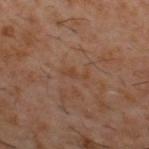Findings:
• follow-up — catalogued during a skin exam; not biopsied
• patient — male, approximately 60 years of age
• site — the back
• image source — 15 mm crop, total-body photography
• tile lighting — cross-polarized illumination